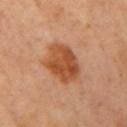- notes · catalogued during a skin exam; not biopsied
- patient · female, about 35 years old
- anatomic site · the right upper arm
- illumination · cross-polarized illumination
- image-analysis metrics · a footprint of about 15 mm², a shape eccentricity near 0.55, and a symmetry-axis asymmetry near 0.15
- image · 15 mm crop, total-body photography